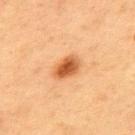diameter = ≈3.5 mm | anatomic site = the upper back | tile lighting = cross-polarized illumination | TBP lesion metrics = a mean CIELAB color near L≈52 a*≈26 b*≈40 and a normalized border contrast of about 10; a lesion-detection confidence of about 100/100 | patient = male, in their mid-50s | acquisition = ~15 mm tile from a whole-body skin photo.